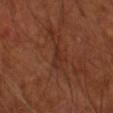subject = male, about 65 years old; image source = ~15 mm tile from a whole-body skin photo; automated lesion analysis = an automated nevus-likeness rating near 0 out of 100 and a lesion-detection confidence of about 55/100; diameter = ≈3 mm; body site = the right forearm; tile lighting = cross-polarized.